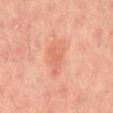Recorded during total-body skin imaging; not selected for excision or biopsy. A region of skin cropped from a whole-body photographic capture, roughly 15 mm wide. A male patient, aged 43–47. On the back.